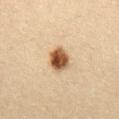Clinical impression: Recorded during total-body skin imaging; not selected for excision or biopsy. Clinical summary: The tile uses cross-polarized illumination. This image is a 15 mm lesion crop taken from a total-body photograph. The lesion is located on the lower back. A female subject in their 30s. The recorded lesion diameter is about 3 mm.